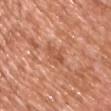Q: Was this lesion biopsied?
A: total-body-photography surveillance lesion; no biopsy
Q: What kind of image is this?
A: ~15 mm crop, total-body skin-cancer survey
Q: Patient demographics?
A: male, approximately 45 years of age
Q: What lighting was used for the tile?
A: white-light
Q: What did automated image analysis measure?
A: an area of roughly 4 mm², an eccentricity of roughly 0.9, and two-axis asymmetry of about 0.25; an average lesion color of about L≈55 a*≈27 b*≈35 (CIELAB) and a lesion-to-skin contrast of about 6 (normalized; higher = more distinct); a nevus-likeness score of about 0/100
Q: Lesion location?
A: the chest
Q: How large is the lesion?
A: ≈3 mm